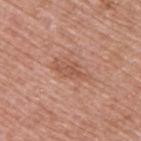  biopsy_status: not biopsied; imaged during a skin examination
  image:
    source: total-body photography crop
    field_of_view_mm: 15
  lesion_size:
    long_diameter_mm_approx: 4.5
  patient:
    sex: male
    age_approx: 70
  site: upper back
  lighting: white-light